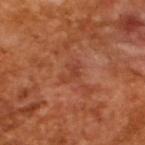This lesion was catalogued during total-body skin photography and was not selected for biopsy. Imaged with cross-polarized lighting. The lesion-visualizer software estimated a lesion area of about 3.5 mm² and a shape eccentricity near 0.8. The software also gave a within-lesion color-variation index near 1/10 and radial color variation of about 0. The analysis additionally found a lesion-detection confidence of about 100/100. A close-up tile cropped from a whole-body skin photograph, about 15 mm across. The lesion's longest dimension is about 3 mm. A male subject, about 65 years old.